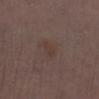The lesion was tiled from a total-body skin photograph and was not biopsied.
Imaged with white-light lighting.
Approximately 2.5 mm at its widest.
Automated tile analysis of the lesion measured a shape eccentricity near 0.85 and a shape-asymmetry score of about 0.3 (0 = symmetric). And it measured a lesion color around L≈37 a*≈15 b*≈20 in CIELAB and a normalized lesion–skin contrast near 4.5. The analysis additionally found border irregularity of about 3 on a 0–10 scale and internal color variation of about 0.5 on a 0–10 scale. And it measured an automated nevus-likeness rating near 5 out of 100.
The lesion is located on the left lower leg.
A region of skin cropped from a whole-body photographic capture, roughly 15 mm wide.
The subject is a male in their 70s.